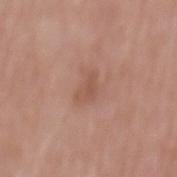biopsy status: no biopsy performed (imaged during a skin exam)
location: the back
illumination: white-light
image: total-body-photography crop, ~15 mm field of view
size: ~3 mm (longest diameter)
subject: male, in their mid- to late 70s
automated lesion analysis: two-axis asymmetry of about 0.45; internal color variation of about 1 on a 0–10 scale and peripheral color asymmetry of about 0.5